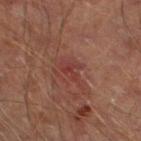biopsy status = no biopsy performed (imaged during a skin exam) | site = the leg | patient = male, roughly 65 years of age | imaging modality = 15 mm crop, total-body photography.